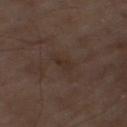<record>
  <biopsy_status>not biopsied; imaged during a skin examination</biopsy_status>
  <lighting>cross-polarized</lighting>
  <automated_metrics>
    <area_mm2_approx>3.5</area_mm2_approx>
    <eccentricity>0.85</eccentricity>
    <shape_asymmetry>0.4</shape_asymmetry>
    <cielab_L>29</cielab_L>
    <cielab_a>15</cielab_a>
    <cielab_b>21</cielab_b>
    <vs_skin_contrast_norm>5.0</vs_skin_contrast_norm>
    <border_irregularity_0_10>4.0</border_irregularity_0_10>
    <color_variation_0_10>1.0</color_variation_0_10>
    <peripheral_color_asymmetry>0.5</peripheral_color_asymmetry>
  </automated_metrics>
  <lesion_size>
    <long_diameter_mm_approx>3.0</long_diameter_mm_approx>
  </lesion_size>
  <site>right thigh</site>
  <image>
    <source>total-body photography crop</source>
    <field_of_view_mm>15</field_of_view_mm>
  </image>
</record>